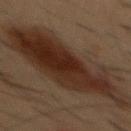Assessment: The lesion was tiled from a total-body skin photograph and was not biopsied. Background: The total-body-photography lesion software estimated an area of roughly 60 mm², an eccentricity of roughly 0.9, and a shape-asymmetry score of about 0.25 (0 = symmetric). From the chest. A 15 mm close-up extracted from a 3D total-body photography capture. The subject is a male about 55 years old. The tile uses cross-polarized illumination. Approximately 14 mm at its widest.